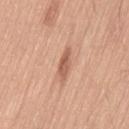Clinical impression:
The lesion was photographed on a routine skin check and not biopsied; there is no pathology result.
Image and clinical context:
The patient is a male roughly 35 years of age. Approximately 4 mm at its widest. The lesion is located on the left thigh. This image is a 15 mm lesion crop taken from a total-body photograph.